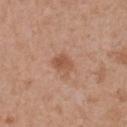follow-up: total-body-photography surveillance lesion; no biopsy
image source: ~15 mm crop, total-body skin-cancer survey
site: the upper back
automated lesion analysis: about 9 CIELAB-L* units darker than the surrounding skin and a normalized border contrast of about 6.5; a border-irregularity index near 2.5/10; an automated nevus-likeness rating near 30 out of 100 and a detector confidence of about 100 out of 100 that the crop contains a lesion
patient: female, aged 38 to 42
size: ≈2.5 mm
tile lighting: white-light illumination